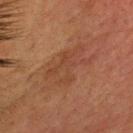Clinical impression: Captured during whole-body skin photography for melanoma surveillance; the lesion was not biopsied. Clinical summary: A male patient in their 60s. The lesion is on the head or neck. Automated tile analysis of the lesion measured a lesion area of about 16 mm² and a shape eccentricity near 0.75. The analysis additionally found a lesion color around L≈36 a*≈19 b*≈27 in CIELAB, about 5 CIELAB-L* units darker than the surrounding skin, and a normalized border contrast of about 4.5. Approximately 6 mm at its widest. A 15 mm crop from a total-body photograph taken for skin-cancer surveillance.